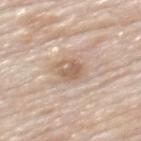follow-up: catalogued during a skin exam; not biopsied
lighting: white-light illumination
anatomic site: the upper back
image-analysis metrics: a lesion color around L≈61 a*≈16 b*≈29 in CIELAB and about 10 CIELAB-L* units darker than the surrounding skin; a border-irregularity index near 2/10, a color-variation rating of about 5/10, and peripheral color asymmetry of about 2; a detector confidence of about 100 out of 100 that the crop contains a lesion
subject: male, aged around 85 or older
imaging modality: total-body-photography crop, ~15 mm field of view